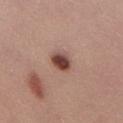This lesion was catalogued during total-body skin photography and was not selected for biopsy. The tile uses white-light illumination. A male subject, about 30 years old. Automated tile analysis of the lesion measured a footprint of about 5.5 mm², an outline eccentricity of about 0.65 (0 = round, 1 = elongated), and two-axis asymmetry of about 0.1. The analysis additionally found a mean CIELAB color near L≈45 a*≈22 b*≈25, about 16 CIELAB-L* units darker than the surrounding skin, and a normalized lesion–skin contrast near 11.5. And it measured a color-variation rating of about 4/10. A region of skin cropped from a whole-body photographic capture, roughly 15 mm wide. Approximately 3 mm at its widest.